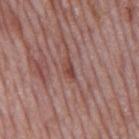biopsy status=no biopsy performed (imaged during a skin exam)
location=the mid back
automated lesion analysis=a lesion area of about 2.5 mm² and a symmetry-axis asymmetry near 0.4; a mean CIELAB color near L≈44 a*≈23 b*≈24 and a lesion–skin lightness drop of about 9; a border-irregularity rating of about 4/10, a color-variation rating of about 2.5/10, and a peripheral color-asymmetry measure near 1; a nevus-likeness score of about 0/100 and a detector confidence of about 90 out of 100 that the crop contains a lesion
size=≈3 mm
imaging modality=15 mm crop, total-body photography
subject=male, in their mid- to late 70s
illumination=white-light